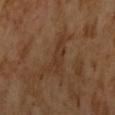A male subject aged approximately 65. A lesion tile, about 15 mm wide, cut from a 3D total-body photograph. Imaged with cross-polarized lighting.